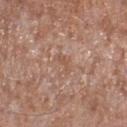Clinical impression: This lesion was catalogued during total-body skin photography and was not selected for biopsy. Clinical summary: A male subject aged approximately 55. Located on the right lower leg. Measured at roughly 2.5 mm in maximum diameter. Captured under white-light illumination. Cropped from a whole-body photographic skin survey; the tile spans about 15 mm.